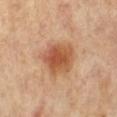Q: Is there a histopathology result?
A: imaged on a skin check; not biopsied
Q: What are the patient's age and sex?
A: female, aged 63–67
Q: What is the anatomic site?
A: the right leg
Q: What is the lesion's diameter?
A: about 4.5 mm
Q: Illumination type?
A: cross-polarized illumination
Q: What kind of image is this?
A: ~15 mm crop, total-body skin-cancer survey